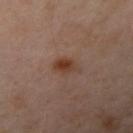• biopsy status — no biopsy performed (imaged during a skin exam)
• imaging modality — ~15 mm crop, total-body skin-cancer survey
• subject — female, aged approximately 40
• location — the arm
• lesion size — about 3 mm
• image-analysis metrics — a mean CIELAB color near L≈39 a*≈20 b*≈28, a lesion–skin lightness drop of about 9, and a normalized lesion–skin contrast near 9; border irregularity of about 3 on a 0–10 scale, a within-lesion color-variation index near 4/10, and radial color variation of about 1.5; a detector confidence of about 100 out of 100 that the crop contains a lesion